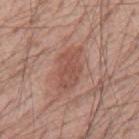Recorded during total-body skin imaging; not selected for excision or biopsy.
The tile uses white-light illumination.
On the mid back.
A 15 mm close-up extracted from a 3D total-body photography capture.
The subject is a male about 55 years old.
Automated tile analysis of the lesion measured an area of roughly 11 mm², an eccentricity of roughly 0.85, and two-axis asymmetry of about 0.3. The software also gave border irregularity of about 3.5 on a 0–10 scale, a within-lesion color-variation index near 2.5/10, and a peripheral color-asymmetry measure near 1. The analysis additionally found a nevus-likeness score of about 85/100 and a lesion-detection confidence of about 100/100.
About 5 mm across.